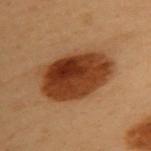Case summary:
* notes: imaged on a skin check; not biopsied
* tile lighting: cross-polarized
* patient: female, aged 58 to 62
* image source: total-body-photography crop, ~15 mm field of view
* image-analysis metrics: a lesion area of about 30 mm², an eccentricity of roughly 0.85, and a shape-asymmetry score of about 0.1 (0 = symmetric); a border-irregularity rating of about 1.5/10, a color-variation rating of about 6.5/10, and a peripheral color-asymmetry measure near 2.5; a lesion-detection confidence of about 100/100
* body site: the back
* size: about 8.5 mm